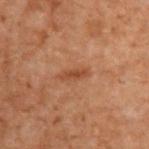The lesion was tiled from a total-body skin photograph and was not biopsied. Cropped from a whole-body photographic skin survey; the tile spans about 15 mm. Captured under cross-polarized illumination. On the upper back. A male patient aged around 70.